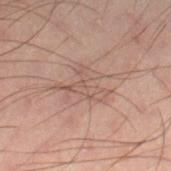Case summary:
– workup · catalogued during a skin exam; not biopsied
– anatomic site · the right lower leg
– patient · male, about 40 years old
– acquisition · ~15 mm crop, total-body skin-cancer survey
– lighting · cross-polarized
– image-analysis metrics · a footprint of about 8.5 mm² and an outline eccentricity of about 0.7 (0 = round, 1 = elongated); a nevus-likeness score of about 0/100 and a detector confidence of about 90 out of 100 that the crop contains a lesion